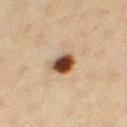  automated_metrics:
    cielab_L: 40
    cielab_a: 17
    cielab_b: 28
    vs_skin_darker_L: 20.0
    vs_skin_contrast_norm: 14.5
    border_irregularity_0_10: 2.0
    color_variation_0_10: 9.5
    peripheral_color_asymmetry: 3.0
    nevus_likeness_0_100: 100
    lesion_detection_confidence_0_100: 100
  site: right thigh
  patient:
    sex: female
    age_approx: 40
  lesion_size:
    long_diameter_mm_approx: 3.5
  lighting: cross-polarized
  image:
    source: total-body photography crop
    field_of_view_mm: 15